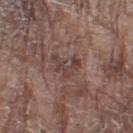Clinical impression:
Part of a total-body skin-imaging series; this lesion was reviewed on a skin check and was not flagged for biopsy.
Image and clinical context:
A roughly 15 mm field-of-view crop from a total-body skin photograph. From the right thigh. Longest diameter approximately 3.5 mm. A male subject aged 78 to 82. An algorithmic analysis of the crop reported a shape eccentricity near 0.7 and a shape-asymmetry score of about 0.6 (0 = symmetric). The software also gave a lesion color around L≈41 a*≈17 b*≈19 in CIELAB, about 8 CIELAB-L* units darker than the surrounding skin, and a normalized lesion–skin contrast near 6.5. It also reported a border-irregularity index near 8/10 and radial color variation of about 0. And it measured lesion-presence confidence of about 65/100.